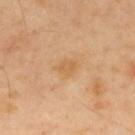| field | value |
|---|---|
| subject | male, aged 38–42 |
| acquisition | 15 mm crop, total-body photography |
| site | the upper back |